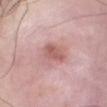Clinical impression: The lesion was photographed on a routine skin check and not biopsied; there is no pathology result.